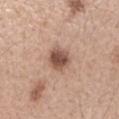The lesion was photographed on a routine skin check and not biopsied; there is no pathology result. A lesion tile, about 15 mm wide, cut from a 3D total-body photograph. On the left forearm. Automated image analysis of the tile measured a footprint of about 6.5 mm², an eccentricity of roughly 0.4, and two-axis asymmetry of about 0.15. Approximately 3 mm at its widest. The subject is a female in their mid-40s. Imaged with white-light lighting.